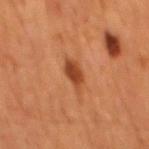Clinical impression:
The lesion was tiled from a total-body skin photograph and was not biopsied.
Image and clinical context:
A male patient, approximately 65 years of age. From the mid back. A close-up tile cropped from a whole-body skin photograph, about 15 mm across.